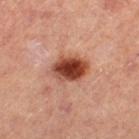Part of a total-body skin-imaging series; this lesion was reviewed on a skin check and was not flagged for biopsy. Automated tile analysis of the lesion measured a border-irregularity rating of about 1.5/10, a within-lesion color-variation index near 7.5/10, and peripheral color asymmetry of about 2. And it measured a nevus-likeness score of about 100/100 and lesion-presence confidence of about 100/100. A female subject, aged 43 to 47. The lesion is located on the left thigh. The tile uses cross-polarized illumination. This image is a 15 mm lesion crop taken from a total-body photograph. The recorded lesion diameter is about 4.5 mm.